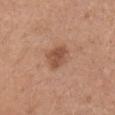Q: Was this lesion biopsied?
A: no biopsy performed (imaged during a skin exam)
Q: What is the imaging modality?
A: ~15 mm crop, total-body skin-cancer survey
Q: What are the patient's age and sex?
A: female, in their mid- to late 50s
Q: Lesion location?
A: the head or neck